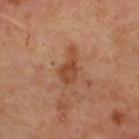Assessment: The lesion was tiled from a total-body skin photograph and was not biopsied. Image and clinical context: This is a cross-polarized tile. Cropped from a whole-body photographic skin survey; the tile spans about 15 mm. A male patient, aged approximately 55. From the upper back.